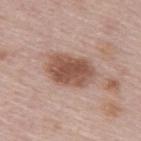subject — female, aged around 65 | imaging modality — ~15 mm tile from a whole-body skin photo | site — the upper back.